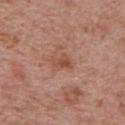| field | value |
|---|---|
| workup | no biopsy performed (imaged during a skin exam) |
| lighting | white-light illumination |
| automated lesion analysis | radial color variation of about 1 |
| size | ~2.5 mm (longest diameter) |
| patient | male, in their 70s |
| image source | ~15 mm crop, total-body skin-cancer survey |
| site | the chest |